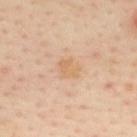patient — female, aged approximately 40 | illumination — cross-polarized illumination | automated metrics — a lesion area of about 5.5 mm², an eccentricity of roughly 0.5, and a shape-asymmetry score of about 0.25 (0 = symmetric); a mean CIELAB color near L≈68 a*≈19 b*≈36 and a lesion-to-skin contrast of about 5 (normalized; higher = more distinct); a color-variation rating of about 2.5/10 and peripheral color asymmetry of about 1 | size — ~2.5 mm (longest diameter) | acquisition — total-body-photography crop, ~15 mm field of view | site — the upper back.